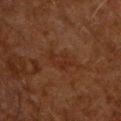<lesion>
  <biopsy_status>not biopsied; imaged during a skin examination</biopsy_status>
  <lighting>cross-polarized</lighting>
  <patient>
    <sex>male</sex>
    <age_approx>60</age_approx>
  </patient>
  <lesion_size>
    <long_diameter_mm_approx>3.5</long_diameter_mm_approx>
  </lesion_size>
  <site>chest</site>
  <image>
    <source>total-body photography crop</source>
    <field_of_view_mm>15</field_of_view_mm>
  </image>
  <automated_metrics>
    <cielab_L>21</cielab_L>
    <cielab_a>18</cielab_a>
    <cielab_b>23</cielab_b>
    <vs_skin_darker_L>4.0</vs_skin_darker_L>
  </automated_metrics>
</lesion>